On the right lower leg. Imaged with white-light lighting. A 15 mm close-up extracted from a 3D total-body photography capture. A female subject, about 65 years old. Longest diameter approximately 2 mm: 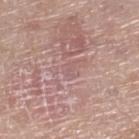Q: What did the biopsy show?
A: an actinic keratosis (borderline)